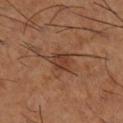Impression: Recorded during total-body skin imaging; not selected for excision or biopsy. Context: A male patient in their mid- to late 60s. Automated tile analysis of the lesion measured a footprint of about 6.5 mm² and a symmetry-axis asymmetry near 0.25. The software also gave an average lesion color of about L≈39 a*≈21 b*≈30 (CIELAB), a lesion–skin lightness drop of about 8, and a lesion-to-skin contrast of about 7 (normalized; higher = more distinct). A 15 mm close-up tile from a total-body photography series done for melanoma screening. On the right lower leg. Captured under cross-polarized illumination.